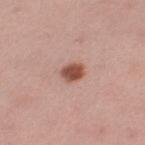  biopsy_status: not biopsied; imaged during a skin examination
  site: left thigh
  lesion_size:
    long_diameter_mm_approx: 2.5
  image:
    source: total-body photography crop
    field_of_view_mm: 15
  lighting: white-light
  patient:
    sex: female
    age_approx: 35